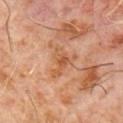Notes:
- notes · catalogued during a skin exam; not biopsied
- anatomic site · the chest
- illumination · cross-polarized
- TBP lesion metrics · a lesion color around L≈52 a*≈23 b*≈35 in CIELAB and about 7 CIELAB-L* units darker than the surrounding skin; a classifier nevus-likeness of about 0/100 and lesion-presence confidence of about 100/100
- patient · male, aged 58–62
- acquisition · 15 mm crop, total-body photography